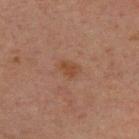The lesion was photographed on a routine skin check and not biopsied; there is no pathology result. The subject is a male aged 28–32. Approximately 2.5 mm at its widest. An algorithmic analysis of the crop reported a lesion color around L≈37 a*≈18 b*≈27 in CIELAB. It also reported a detector confidence of about 100 out of 100 that the crop contains a lesion. A close-up tile cropped from a whole-body skin photograph, about 15 mm across. The lesion is located on the upper back.